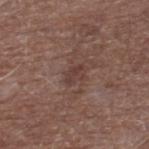notes: imaged on a skin check; not biopsied | automated lesion analysis: an area of roughly 3 mm², a shape eccentricity near 0.8, and a symmetry-axis asymmetry near 0.3; an average lesion color of about L≈38 a*≈19 b*≈22 (CIELAB), a lesion–skin lightness drop of about 7, and a normalized lesion–skin contrast near 6; a detector confidence of about 100 out of 100 that the crop contains a lesion | site: the right lower leg | image source: 15 mm crop, total-body photography | illumination: white-light illumination | subject: male, aged 68–72.